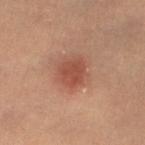Clinical impression:
No biopsy was performed on this lesion — it was imaged during a full skin examination and was not determined to be concerning.
Clinical summary:
A female patient, aged 33–37. The lesion is located on the leg. Cropped from a whole-body photographic skin survey; the tile spans about 15 mm. This is a cross-polarized tile. The recorded lesion diameter is about 3 mm.